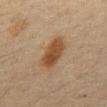{"biopsy_status": "not biopsied; imaged during a skin examination", "image": {"source": "total-body photography crop", "field_of_view_mm": 15}, "lighting": "cross-polarized", "patient": {"sex": "male", "age_approx": 65}, "site": "mid back"}